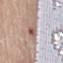The lesion was tiled from a total-body skin photograph and was not biopsied.
A 15 mm close-up extracted from a 3D total-body photography capture.
A female subject, aged 68–72.
The lesion is located on the mid back.